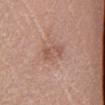{"biopsy_status": "not biopsied; imaged during a skin examination", "site": "left lower leg", "lighting": "white-light", "lesion_size": {"long_diameter_mm_approx": 4.0}, "automated_metrics": {"area_mm2_approx": 6.0, "eccentricity": 0.9, "shape_asymmetry": 0.25, "nevus_likeness_0_100": 0, "lesion_detection_confidence_0_100": 100}, "patient": {"sex": "female", "age_approx": 35}, "image": {"source": "total-body photography crop", "field_of_view_mm": 15}}